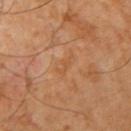Q: How large is the lesion?
A: about 3 mm
Q: Where on the body is the lesion?
A: the right upper arm
Q: Automated lesion metrics?
A: a shape eccentricity near 0.95 and a shape-asymmetry score of about 0.35 (0 = symmetric); a lesion-to-skin contrast of about 4.5 (normalized; higher = more distinct); a nevus-likeness score of about 0/100 and a lesion-detection confidence of about 100/100
Q: Who is the patient?
A: male, approximately 60 years of age
Q: Illumination type?
A: cross-polarized illumination
Q: What kind of image is this?
A: ~15 mm crop, total-body skin-cancer survey